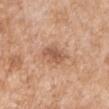<case>
<biopsy_status>not biopsied; imaged during a skin examination</biopsy_status>
<image>
  <source>total-body photography crop</source>
  <field_of_view_mm>15</field_of_view_mm>
</image>
<site>right upper arm</site>
<lesion_size>
  <long_diameter_mm_approx>3.0</long_diameter_mm_approx>
</lesion_size>
<patient>
  <sex>male</sex>
  <age_approx>60</age_approx>
</patient>
<lighting>white-light</lighting>
<automated_metrics>
  <cielab_L>56</cielab_L>
  <cielab_a>22</cielab_a>
  <cielab_b>32</cielab_b>
  <vs_skin_darker_L>10.0</vs_skin_darker_L>
  <vs_skin_contrast_norm>7.0</vs_skin_contrast_norm>
</automated_metrics>
</case>